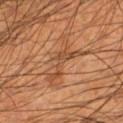Q: Was a biopsy performed?
A: imaged on a skin check; not biopsied
Q: Who is the patient?
A: male, approximately 55 years of age
Q: Where on the body is the lesion?
A: the left lower leg
Q: What is the imaging modality?
A: ~15 mm tile from a whole-body skin photo
Q: How was the tile lit?
A: cross-polarized illumination
Q: Lesion size?
A: ~6 mm (longest diameter)